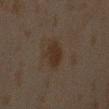| key | value |
|---|---|
| notes | imaged on a skin check; not biopsied |
| location | the arm |
| patient | male, aged approximately 45 |
| acquisition | ~15 mm crop, total-body skin-cancer survey |